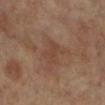patient=female, aged 63 to 67
anatomic site=the left leg
image source=15 mm crop, total-body photography
illumination=cross-polarized